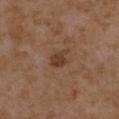The lesion was photographed on a routine skin check and not biopsied; there is no pathology result. A 15 mm close-up extracted from a 3D total-body photography capture. An algorithmic analysis of the crop reported a lesion area of about 4 mm², an outline eccentricity of about 0.75 (0 = round, 1 = elongated), and a symmetry-axis asymmetry near 0.3. The software also gave a mean CIELAB color near L≈39 a*≈19 b*≈28 and about 9 CIELAB-L* units darker than the surrounding skin. It also reported a within-lesion color-variation index near 2/10 and peripheral color asymmetry of about 0.5. The analysis additionally found a classifier nevus-likeness of about 55/100. A female patient about 35 years old. The lesion is on the right upper arm. Measured at roughly 3 mm in maximum diameter.